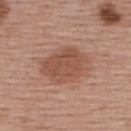No biopsy was performed on this lesion — it was imaged during a full skin examination and was not determined to be concerning.